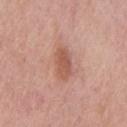Assessment: The lesion was tiled from a total-body skin photograph and was not biopsied. Acquisition and patient details: A male subject, roughly 75 years of age. A region of skin cropped from a whole-body photographic capture, roughly 15 mm wide. The lesion is on the chest.